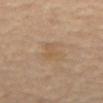<record>
  <biopsy_status>not biopsied; imaged during a skin examination</biopsy_status>
  <lesion_size>
    <long_diameter_mm_approx>2.5</long_diameter_mm_approx>
  </lesion_size>
  <patient>
    <sex>male</sex>
    <age_approx>60</age_approx>
  </patient>
  <image>
    <source>total-body photography crop</source>
    <field_of_view_mm>15</field_of_view_mm>
  </image>
  <lighting>cross-polarized</lighting>
  <automated_metrics>
    <area_mm2_approx>4.5</area_mm2_approx>
    <eccentricity>0.7</eccentricity>
    <shape_asymmetry>0.25</shape_asymmetry>
    <cielab_L>53</cielab_L>
    <cielab_a>14</cielab_a>
    <cielab_b>32</cielab_b>
    <vs_skin_darker_L>5.0</vs_skin_darker_L>
    <vs_skin_contrast_norm>4.5</vs_skin_contrast_norm>
    <nevus_likeness_0_100>0</nevus_likeness_0_100>
    <lesion_detection_confidence_0_100>100</lesion_detection_confidence_0_100>
  </automated_metrics>
  <site>abdomen</site>
</record>